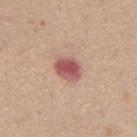Q: Was this lesion biopsied?
A: imaged on a skin check; not biopsied
Q: What is the imaging modality?
A: ~15 mm crop, total-body skin-cancer survey
Q: Lesion location?
A: the mid back
Q: Who is the patient?
A: male, in their mid- to late 60s